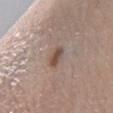Captured during whole-body skin photography for melanoma surveillance; the lesion was not biopsied.